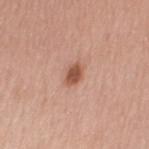– follow-up — imaged on a skin check; not biopsied
– imaging modality — ~15 mm tile from a whole-body skin photo
– patient — female, aged 68–72
– site — the left upper arm